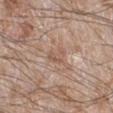* site · the right lower leg
* lighting · white-light
* lesion size · about 2.5 mm
* imaging modality · ~15 mm crop, total-body skin-cancer survey
* automated lesion analysis · a footprint of about 4 mm² and two-axis asymmetry of about 0.45; a border-irregularity index near 4.5/10 and internal color variation of about 2.5 on a 0–10 scale
* patient · male, approximately 60 years of age